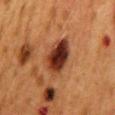Notes:
* biopsy status: total-body-photography surveillance lesion; no biopsy
* image source: 15 mm crop, total-body photography
* lesion size: ≈5 mm
* anatomic site: the mid back
* patient: female, aged approximately 50